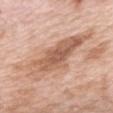| field | value |
|---|---|
| follow-up | catalogued during a skin exam; not biopsied |
| patient | female, roughly 65 years of age |
| image source | ~15 mm crop, total-body skin-cancer survey |
| lighting | white-light |
| TBP lesion metrics | a lesion–skin lightness drop of about 11 and a normalized border contrast of about 7.5; a border-irregularity index near 4.5/10, a within-lesion color-variation index near 5.5/10, and a peripheral color-asymmetry measure near 2; a nevus-likeness score of about 5/100 and lesion-presence confidence of about 100/100 |
| location | the upper back |
| diameter | ≈8.5 mm |